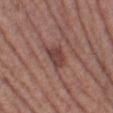Clinical impression: Imaged during a routine full-body skin examination; the lesion was not biopsied and no histopathology is available. Image and clinical context: The tile uses white-light illumination. On the left thigh. A female patient, aged 38–42. A 15 mm close-up tile from a total-body photography series done for melanoma screening.